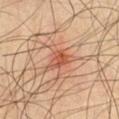| key | value |
|---|---|
| biopsy status | no biopsy performed (imaged during a skin exam) |
| subject | male, in their mid- to late 50s |
| tile lighting | cross-polarized |
| TBP lesion metrics | a border-irregularity index near 3/10 and peripheral color asymmetry of about 2 |
| image | ~15 mm crop, total-body skin-cancer survey |
| body site | the chest |